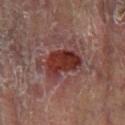| field | value |
|---|---|
| follow-up | no biopsy performed (imaged during a skin exam) |